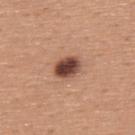Impression:
Captured during whole-body skin photography for melanoma surveillance; the lesion was not biopsied.
Background:
Automated image analysis of the tile measured a shape eccentricity near 0.7 and a symmetry-axis asymmetry near 0.2. The software also gave an average lesion color of about L≈46 a*≈22 b*≈27 (CIELAB) and a lesion–skin lightness drop of about 17. The software also gave an automated nevus-likeness rating near 90 out of 100 and a detector confidence of about 100 out of 100 that the crop contains a lesion. A male subject aged around 45. Located on the upper back. This image is a 15 mm lesion crop taken from a total-body photograph.